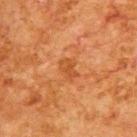biopsy status = total-body-photography surveillance lesion; no biopsy | image source = ~15 mm crop, total-body skin-cancer survey | subject = male, about 80 years old | site = the upper back.